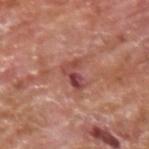Notes:
* biopsy status — total-body-photography surveillance lesion; no biopsy
* body site — the upper back
* image — 15 mm crop, total-body photography
* lighting — white-light
* image-analysis metrics — a border-irregularity rating of about 5.5/10 and radial color variation of about 4; a detector confidence of about 95 out of 100 that the crop contains a lesion
* subject — male, aged approximately 65